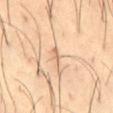Assessment: No biopsy was performed on this lesion — it was imaged during a full skin examination and was not determined to be concerning. Context: A 15 mm crop from a total-body photograph taken for skin-cancer surveillance. From the abdomen. A male patient, aged 38–42. Imaged with cross-polarized lighting.